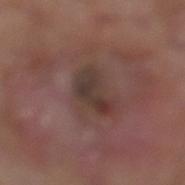Recorded during total-body skin imaging; not selected for excision or biopsy. A male patient, roughly 80 years of age. Imaged with white-light lighting. The recorded lesion diameter is about 4.5 mm. The total-body-photography lesion software estimated a lesion area of about 8 mm², an eccentricity of roughly 0.85, and two-axis asymmetry of about 0.45. And it measured an automated nevus-likeness rating near 0 out of 100. On the left lower leg. Cropped from a whole-body photographic skin survey; the tile spans about 15 mm.